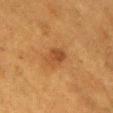{
  "biopsy_status": "not biopsied; imaged during a skin examination",
  "patient": {
    "sex": "female",
    "age_approx": 40
  },
  "image": {
    "source": "total-body photography crop",
    "field_of_view_mm": 15
  },
  "site": "chest"
}